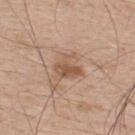patient: male, aged 48–52
image source: ~15 mm crop, total-body skin-cancer survey
location: the upper back